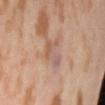workup: imaged on a skin check; not biopsied
TBP lesion metrics: a mean CIELAB color near L≈58 a*≈19 b*≈27 and a lesion-to-skin contrast of about 5 (normalized; higher = more distinct); border irregularity of about 5 on a 0–10 scale, a within-lesion color-variation index near 8/10, and peripheral color asymmetry of about 3; a classifier nevus-likeness of about 0/100 and a detector confidence of about 100 out of 100 that the crop contains a lesion
subject: female, in their mid-50s
image: 15 mm crop, total-body photography
location: the lower back
tile lighting: cross-polarized